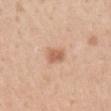This lesion was catalogued during total-body skin photography and was not selected for biopsy.
The tile uses white-light illumination.
A female patient, about 30 years old.
The lesion's longest dimension is about 2.5 mm.
A lesion tile, about 15 mm wide, cut from a 3D total-body photograph.
The lesion is on the chest.